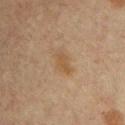Case summary:
– image-analysis metrics — an area of roughly 4.5 mm², an eccentricity of roughly 0.85, and a symmetry-axis asymmetry near 0.25; a lesion–skin lightness drop of about 6 and a lesion-to-skin contrast of about 6 (normalized; higher = more distinct); a border-irregularity index near 2.5/10 and a within-lesion color-variation index near 1/10; a classifier nevus-likeness of about 10/100 and lesion-presence confidence of about 100/100
– lesion size — ≈3 mm
– anatomic site — the chest
– subject — male, in their 60s
– image — ~15 mm crop, total-body skin-cancer survey
– tile lighting — cross-polarized